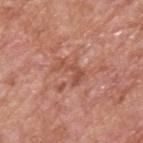Image and clinical context: A male subject aged 63–67. Measured at roughly 3.5 mm in maximum diameter. Captured under white-light illumination. A 15 mm close-up extracted from a 3D total-body photography capture. Located on the upper back.